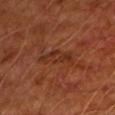Captured during whole-body skin photography for melanoma surveillance; the lesion was not biopsied.